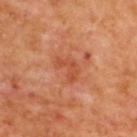Assessment:
Recorded during total-body skin imaging; not selected for excision or biopsy.
Context:
A 15 mm close-up extracted from a 3D total-body photography capture. A male subject roughly 70 years of age. The lesion is on the upper back.